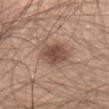Recorded during total-body skin imaging; not selected for excision or biopsy. A female patient approximately 40 years of age. The lesion is on the head or neck. Measured at roughly 4 mm in maximum diameter. This image is a 15 mm lesion crop taken from a total-body photograph. The tile uses white-light illumination. Automated tile analysis of the lesion measured a lesion color around L≈49 a*≈19 b*≈25 in CIELAB and roughly 12 lightness units darker than nearby skin. The analysis additionally found an automated nevus-likeness rating near 75 out of 100 and a detector confidence of about 100 out of 100 that the crop contains a lesion.